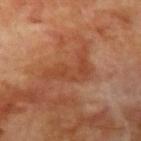| feature | finding |
|---|---|
| workup | imaged on a skin check; not biopsied |
| diameter | ≈6 mm |
| imaging modality | ~15 mm tile from a whole-body skin photo |
| site | the arm |
| patient | male, aged approximately 70 |